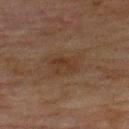Assessment: Part of a total-body skin-imaging series; this lesion was reviewed on a skin check and was not flagged for biopsy. Image and clinical context: A roughly 15 mm field-of-view crop from a total-body skin photograph. Captured under cross-polarized illumination. The lesion's longest dimension is about 2.5 mm. Located on the upper back. The patient is a male aged 68–72.